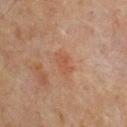{"patient": {"sex": "male", "age_approx": 65}, "site": "upper back", "image": {"source": "total-body photography crop", "field_of_view_mm": 15}}